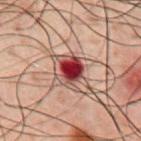Impression:
This lesion was catalogued during total-body skin photography and was not selected for biopsy.
Acquisition and patient details:
A 15 mm close-up extracted from a 3D total-body photography capture. A male subject, aged 58 to 62. From the chest.